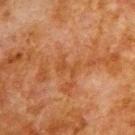– follow-up · no biopsy performed (imaged during a skin exam)
– tile lighting · cross-polarized illumination
– TBP lesion metrics · a normalized border contrast of about 5.5; a border-irregularity index near 7/10 and a within-lesion color-variation index near 0/10; a nevus-likeness score of about 0/100 and a lesion-detection confidence of about 100/100
– anatomic site · the upper back
– patient · male, approximately 80 years of age
– lesion size · ≈2.5 mm
– image · total-body-photography crop, ~15 mm field of view